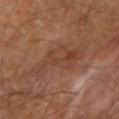Case summary:
• follow-up: total-body-photography surveillance lesion; no biopsy
• location: the right forearm
• patient: male, aged around 70
• lesion diameter: ≈6 mm
• automated lesion analysis: roughly 6 lightness units darker than nearby skin and a normalized border contrast of about 5; a border-irregularity index near 7/10, a color-variation rating of about 4/10, and peripheral color asymmetry of about 1; a classifier nevus-likeness of about 0/100
• tile lighting: cross-polarized
• acquisition: 15 mm crop, total-body photography